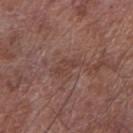Image and clinical context:
A 15 mm crop from a total-body photograph taken for skin-cancer surveillance. The recorded lesion diameter is about 2.5 mm. The subject is a male in their mid- to late 70s. This is a white-light tile. Automated tile analysis of the lesion measured a footprint of about 3.5 mm², an outline eccentricity of about 0.8 (0 = round, 1 = elongated), and a shape-asymmetry score of about 0.4 (0 = symmetric). The analysis additionally found a lesion color around L≈41 a*≈20 b*≈23 in CIELAB and a normalized lesion–skin contrast near 5. And it measured a border-irregularity index near 4/10, a within-lesion color-variation index near 1.5/10, and peripheral color asymmetry of about 0.5. And it measured a classifier nevus-likeness of about 0/100 and a lesion-detection confidence of about 90/100. On the right forearm.